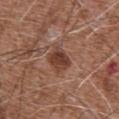Q: Was a biopsy performed?
A: catalogued during a skin exam; not biopsied
Q: What did automated image analysis measure?
A: a shape eccentricity near 0.65 and a symmetry-axis asymmetry near 0.15; a mean CIELAB color near L≈40 a*≈22 b*≈27 and a lesion–skin lightness drop of about 11; border irregularity of about 1.5 on a 0–10 scale, internal color variation of about 3.5 on a 0–10 scale, and radial color variation of about 1.5; an automated nevus-likeness rating near 90 out of 100 and lesion-presence confidence of about 100/100
Q: What is the imaging modality?
A: 15 mm crop, total-body photography
Q: Lesion size?
A: ≈3 mm
Q: What are the patient's age and sex?
A: male, aged approximately 75
Q: Where on the body is the lesion?
A: the abdomen